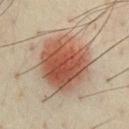Clinical summary: The lesion's longest dimension is about 7 mm. A roughly 15 mm field-of-view crop from a total-body skin photograph. The lesion is on the chest. Automated image analysis of the tile measured a footprint of about 32 mm². And it measured a nevus-likeness score of about 100/100 and a detector confidence of about 100 out of 100 that the crop contains a lesion. A male patient roughly 50 years of age. The tile uses cross-polarized illumination.